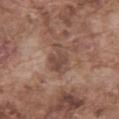Imaged during a routine full-body skin examination; the lesion was not biopsied and no histopathology is available. Imaged with white-light lighting. A region of skin cropped from a whole-body photographic capture, roughly 15 mm wide. Located on the abdomen. The patient is a male aged 73–77.